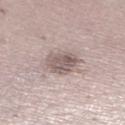No biopsy was performed on this lesion — it was imaged during a full skin examination and was not determined to be concerning. Automated tile analysis of the lesion measured a lesion color around L≈58 a*≈14 b*≈18 in CIELAB and a normalized border contrast of about 7.5. And it measured a border-irregularity index near 1.5/10, a within-lesion color-variation index near 4.5/10, and peripheral color asymmetry of about 1.5. It also reported an automated nevus-likeness rating near 5 out of 100 and a lesion-detection confidence of about 50/100. This is a white-light tile. The lesion is on the right lower leg. A region of skin cropped from a whole-body photographic capture, roughly 15 mm wide. The subject is a female approximately 50 years of age.